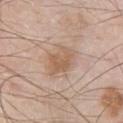Clinical impression: This lesion was catalogued during total-body skin photography and was not selected for biopsy. Context: This image is a 15 mm lesion crop taken from a total-body photograph. Located on the chest. Automated image analysis of the tile measured a lesion area of about 8.5 mm² and a shape eccentricity near 0.65. The analysis additionally found roughly 8 lightness units darker than nearby skin and a normalized border contrast of about 6.5. It also reported a classifier nevus-likeness of about 30/100 and lesion-presence confidence of about 100/100. Captured under white-light illumination. A male patient, about 55 years old. Longest diameter approximately 4 mm.